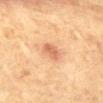Impression: Part of a total-body skin-imaging series; this lesion was reviewed on a skin check and was not flagged for biopsy. Acquisition and patient details: A 15 mm crop from a total-body photograph taken for skin-cancer surveillance. The subject is a male aged around 65. Located on the front of the torso. About 2.5 mm across. The tile uses cross-polarized illumination.